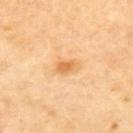Q: How was this image acquired?
A: 15 mm crop, total-body photography
Q: What is the lesion's diameter?
A: ≈3 mm
Q: Where on the body is the lesion?
A: the upper back